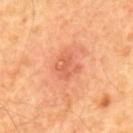No biopsy was performed on this lesion — it was imaged during a full skin examination and was not determined to be concerning. Measured at roughly 3.5 mm in maximum diameter. Located on the mid back. A 15 mm crop from a total-body photograph taken for skin-cancer surveillance. A male patient, aged 63–67.